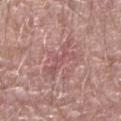Clinical impression:
The lesion was tiled from a total-body skin photograph and was not biopsied.